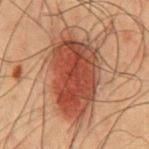* illumination — cross-polarized illumination
* diameter — ~10 mm (longest diameter)
* patient — male, in their 50s
* automated lesion analysis — roughly 12 lightness units darker than nearby skin and a lesion-to-skin contrast of about 10 (normalized; higher = more distinct); a border-irregularity index near 2.5/10, a within-lesion color-variation index near 6/10, and radial color variation of about 2
* image — ~15 mm crop, total-body skin-cancer survey
* site — the front of the torso